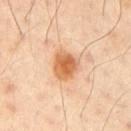{
  "biopsy_status": "not biopsied; imaged during a skin examination",
  "lighting": "cross-polarized",
  "lesion_size": {
    "long_diameter_mm_approx": 3.5
  },
  "image": {
    "source": "total-body photography crop",
    "field_of_view_mm": 15
  },
  "patient": {
    "sex": "male",
    "age_approx": 50
  },
  "site": "left arm"
}